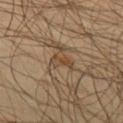– follow-up · imaged on a skin check; not biopsied